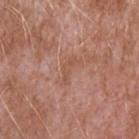| field | value |
|---|---|
| biopsy status | imaged on a skin check; not biopsied |
| illumination | white-light |
| lesion diameter | ~3.5 mm (longest diameter) |
| subject | male, approximately 45 years of age |
| site | the left upper arm |
| image source | total-body-photography crop, ~15 mm field of view |
| TBP lesion metrics | a lesion color around L≈53 a*≈22 b*≈30 in CIELAB, a lesion–skin lightness drop of about 6, and a lesion-to-skin contrast of about 4.5 (normalized; higher = more distinct); a classifier nevus-likeness of about 0/100 and a detector confidence of about 100 out of 100 that the crop contains a lesion |